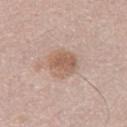Captured during whole-body skin photography for melanoma surveillance; the lesion was not biopsied. Cropped from a total-body skin-imaging series; the visible field is about 15 mm. Imaged with white-light lighting. A male patient aged approximately 30. The lesion's longest dimension is about 3.5 mm.